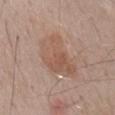follow-up: total-body-photography surveillance lesion; no biopsy
lesion size: ~6.5 mm (longest diameter)
image-analysis metrics: an area of roughly 19 mm², a shape eccentricity near 0.8, and a shape-asymmetry score of about 0.35 (0 = symmetric); roughly 7 lightness units darker than nearby skin and a lesion-to-skin contrast of about 5.5 (normalized; higher = more distinct); a border-irregularity index near 3.5/10, a color-variation rating of about 4/10, and a peripheral color-asymmetry measure near 1.5
tile lighting: white-light illumination
subject: male, aged 53–57
imaging modality: 15 mm crop, total-body photography
site: the mid back